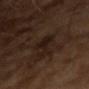follow-up: total-body-photography surveillance lesion; no biopsy | illumination: cross-polarized | size: about 4.5 mm | subject: male, approximately 65 years of age | image-analysis metrics: a footprint of about 5.5 mm², an outline eccentricity of about 0.9 (0 = round, 1 = elongated), and a symmetry-axis asymmetry near 0.45; an average lesion color of about L≈16 a*≈13 b*≈18 (CIELAB), about 6 CIELAB-L* units darker than the surrounding skin, and a normalized lesion–skin contrast near 8.5; a border-irregularity index near 5.5/10, a within-lesion color-variation index near 2/10, and peripheral color asymmetry of about 1 | acquisition: 15 mm crop, total-body photography.